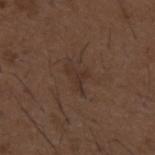Impression:
This lesion was catalogued during total-body skin photography and was not selected for biopsy.
Image and clinical context:
A male subject, approximately 50 years of age. Captured under white-light illumination. A 15 mm close-up tile from a total-body photography series done for melanoma screening. The lesion is on the mid back.